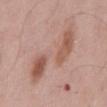Captured during whole-body skin photography for melanoma surveillance; the lesion was not biopsied. A 15 mm close-up tile from a total-body photography series done for melanoma screening. Longest diameter approximately 9.5 mm. The tile uses white-light illumination. From the back. A male patient, aged 53–57.